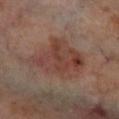| key | value |
|---|---|
| notes | total-body-photography surveillance lesion; no biopsy |
| subject | male, in their 70s |
| lesion size | ~7.5 mm (longest diameter) |
| acquisition | total-body-photography crop, ~15 mm field of view |
| illumination | cross-polarized illumination |
| body site | the right lower leg |